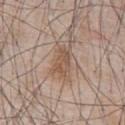The lesion was photographed on a routine skin check and not biopsied; there is no pathology result.
A male patient aged around 65.
This image is a 15 mm lesion crop taken from a total-body photograph.
The lesion is on the chest.
The lesion's longest dimension is about 4 mm.
Imaged with white-light lighting.